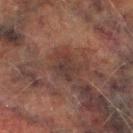Clinical impression:
Part of a total-body skin-imaging series; this lesion was reviewed on a skin check and was not flagged for biopsy.
Context:
The tile uses cross-polarized illumination. A region of skin cropped from a whole-body photographic capture, roughly 15 mm wide. The lesion is on the right lower leg. Automated image analysis of the tile measured a mean CIELAB color near L≈29 a*≈15 b*≈18 and a normalized lesion–skin contrast near 6.5. It also reported a border-irregularity index near 4/10, internal color variation of about 3 on a 0–10 scale, and radial color variation of about 1. A male patient approximately 75 years of age.